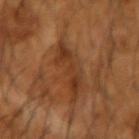• notes — total-body-photography surveillance lesion; no biopsy
• location — the left forearm
• subject — male, aged around 65
• illumination — cross-polarized
• image — total-body-photography crop, ~15 mm field of view
• size — ~6 mm (longest diameter)